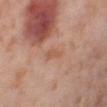notes — imaged on a skin check; not biopsied | site — the right thigh | lesion diameter — about 4 mm | image source — total-body-photography crop, ~15 mm field of view | subject — female, roughly 55 years of age | lighting — cross-polarized illumination.